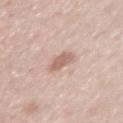Impression:
Imaged during a routine full-body skin examination; the lesion was not biopsied and no histopathology is available.
Background:
The lesion is located on the left upper arm. This is a white-light tile. The total-body-photography lesion software estimated a peripheral color-asymmetry measure near 0.5. It also reported a nevus-likeness score of about 40/100 and a lesion-detection confidence of about 100/100. A region of skin cropped from a whole-body photographic capture, roughly 15 mm wide. The recorded lesion diameter is about 2.5 mm. The patient is a female in their 50s.